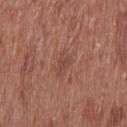biopsy status = catalogued during a skin exam; not biopsied | illumination = white-light | site = the upper back | patient = male, in their mid-70s | image = total-body-photography crop, ~15 mm field of view.